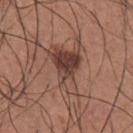Clinical impression: Captured during whole-body skin photography for melanoma surveillance; the lesion was not biopsied. Clinical summary: The patient is a male aged 33 to 37. Located on the chest. The recorded lesion diameter is about 6.5 mm. Captured under white-light illumination. A 15 mm crop from a total-body photograph taken for skin-cancer surveillance.